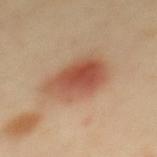Recorded during total-body skin imaging; not selected for excision or biopsy.
Located on the mid back.
Captured under cross-polarized illumination.
Automated tile analysis of the lesion measured a shape eccentricity near 0.75 and two-axis asymmetry of about 0.25. It also reported border irregularity of about 2.5 on a 0–10 scale and a peripheral color-asymmetry measure near 2. The software also gave a classifier nevus-likeness of about 100/100.
A 15 mm close-up extracted from a 3D total-body photography capture.
Approximately 6 mm at its widest.
A female patient about 40 years old.